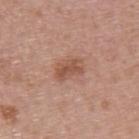follow-up: total-body-photography surveillance lesion; no biopsy
image: ~15 mm crop, total-body skin-cancer survey
subject: male, roughly 55 years of age
lesion diameter: ≈3.5 mm
lighting: white-light illumination